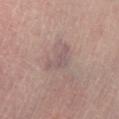Impression:
Imaged during a routine full-body skin examination; the lesion was not biopsied and no histopathology is available.
Context:
From the left lower leg. Imaged with cross-polarized lighting. This image is a 15 mm lesion crop taken from a total-body photograph. Approximately 4 mm at its widest. The patient is a female in their mid- to late 50s.